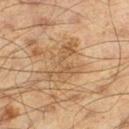This lesion was catalogued during total-body skin photography and was not selected for biopsy. A male subject approximately 60 years of age. On the left thigh. Cropped from a whole-body photographic skin survey; the tile spans about 15 mm.